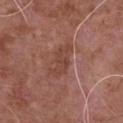Assessment: This lesion was catalogued during total-body skin photography and was not selected for biopsy. Acquisition and patient details: Captured under white-light illumination. A 15 mm close-up extracted from a 3D total-body photography capture. Approximately 4 mm at its widest. On the chest. Automated tile analysis of the lesion measured an outline eccentricity of about 0.9 (0 = round, 1 = elongated) and a shape-asymmetry score of about 0.5 (0 = symmetric). It also reported a lesion color around L≈44 a*≈23 b*≈27 in CIELAB. The analysis additionally found border irregularity of about 5.5 on a 0–10 scale and a color-variation rating of about 2/10. The patient is a male about 75 years old.